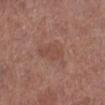  biopsy_status: not biopsied; imaged during a skin examination
  lesion_size:
    long_diameter_mm_approx: 4.5
  lighting: white-light
  site: left lower leg
  image:
    source: total-body photography crop
    field_of_view_mm: 15
  patient:
    sex: female
    age_approx: 50